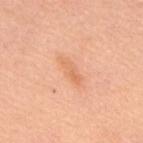Imaged during a routine full-body skin examination; the lesion was not biopsied and no histopathology is available. A male patient, in their mid-60s. The lesion-visualizer software estimated an area of roughly 4.5 mm², an eccentricity of roughly 0.95, and two-axis asymmetry of about 0.25. And it measured a mean CIELAB color near L≈69 a*≈26 b*≈39 and a lesion–skin lightness drop of about 7. It also reported border irregularity of about 3 on a 0–10 scale and a peripheral color-asymmetry measure near 1. It also reported a lesion-detection confidence of about 100/100. Imaged with white-light lighting. The lesion is located on the back. The recorded lesion diameter is about 4 mm. A 15 mm close-up tile from a total-body photography series done for melanoma screening.